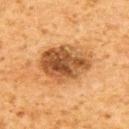Recorded during total-body skin imaging; not selected for excision or biopsy.
The lesion's longest dimension is about 7 mm.
The tile uses cross-polarized illumination.
A male patient in their 60s.
Located on the back.
Cropped from a whole-body photographic skin survey; the tile spans about 15 mm.
An algorithmic analysis of the crop reported a footprint of about 25 mm², a shape eccentricity near 0.75, and a symmetry-axis asymmetry near 0.15. It also reported an automated nevus-likeness rating near 70 out of 100 and a lesion-detection confidence of about 100/100.